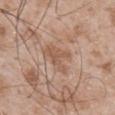workup: catalogued during a skin exam; not biopsied | subject: male, roughly 50 years of age | image: 15 mm crop, total-body photography | tile lighting: white-light illumination | TBP lesion metrics: a lesion area of about 6 mm² and a symmetry-axis asymmetry near 0.55; a mean CIELAB color near L≈55 a*≈19 b*≈30 and a normalized border contrast of about 6; a border-irregularity rating of about 7/10, internal color variation of about 2 on a 0–10 scale, and a peripheral color-asymmetry measure near 0.5; a classifier nevus-likeness of about 0/100 and a lesion-detection confidence of about 100/100 | site: the mid back.